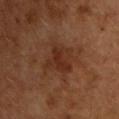| feature | finding |
|---|---|
| notes | catalogued during a skin exam; not biopsied |
| size | ~4 mm (longest diameter) |
| anatomic site | the chest |
| tile lighting | cross-polarized illumination |
| image source | total-body-photography crop, ~15 mm field of view |
| subject | male, aged 48 to 52 |